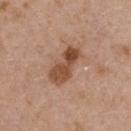Recorded during total-body skin imaging; not selected for excision or biopsy.
A lesion tile, about 15 mm wide, cut from a 3D total-body photograph.
The total-body-photography lesion software estimated a border-irregularity rating of about 3/10, internal color variation of about 6.5 on a 0–10 scale, and peripheral color asymmetry of about 2.5. And it measured an automated nevus-likeness rating near 40 out of 100 and a detector confidence of about 100 out of 100 that the crop contains a lesion.
Located on the chest.
The subject is a female aged 33–37.